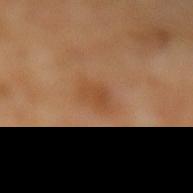* biopsy status: total-body-photography surveillance lesion; no biopsy
* size: ≈3 mm
* subject: female, about 50 years old
* imaging modality: total-body-photography crop, ~15 mm field of view
* tile lighting: cross-polarized
* site: the left lower leg